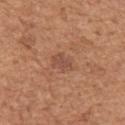• workup · imaged on a skin check; not biopsied
• subject · female, approximately 55 years of age
• body site · the arm
• tile lighting · white-light illumination
• size · ~2.5 mm (longest diameter)
• acquisition · 15 mm crop, total-body photography